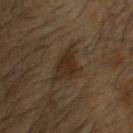No biopsy was performed on this lesion — it was imaged during a full skin examination and was not determined to be concerning.
This image is a 15 mm lesion crop taken from a total-body photograph.
Imaged with cross-polarized lighting.
The lesion is located on the head or neck.
The recorded lesion diameter is about 4.5 mm.
A male subject about 55 years old.